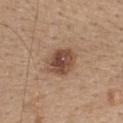Recorded during total-body skin imaging; not selected for excision or biopsy. A 15 mm close-up tile from a total-body photography series done for melanoma screening. An algorithmic analysis of the crop reported a nevus-likeness score of about 80/100 and a detector confidence of about 100 out of 100 that the crop contains a lesion. The tile uses white-light illumination. Approximately 4 mm at its widest. A female subject aged approximately 45. The lesion is located on the upper back.